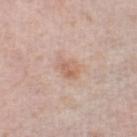Impression:
No biopsy was performed on this lesion — it was imaged during a full skin examination and was not determined to be concerning.
Acquisition and patient details:
The patient is a female aged 58–62. A 15 mm close-up extracted from a 3D total-body photography capture. Captured under white-light illumination. Automated tile analysis of the lesion measured an average lesion color of about L≈62 a*≈19 b*≈29 (CIELAB), about 8 CIELAB-L* units darker than the surrounding skin, and a normalized border contrast of about 6. The software also gave an automated nevus-likeness rating near 15 out of 100 and a lesion-detection confidence of about 100/100. Located on the leg.